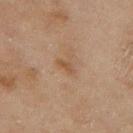No biopsy was performed on this lesion — it was imaged during a full skin examination and was not determined to be concerning.
From the left thigh.
The patient is a female roughly 60 years of age.
This image is a 15 mm lesion crop taken from a total-body photograph.